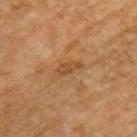This lesion was catalogued during total-body skin photography and was not selected for biopsy. The recorded lesion diameter is about 3 mm. The lesion is located on the left upper arm. This image is a 15 mm lesion crop taken from a total-body photograph. A male patient, aged 53–57.